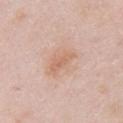A female patient about 65 years old. On the right upper arm. A 15 mm close-up tile from a total-body photography series done for melanoma screening. Measured at roughly 4.5 mm in maximum diameter. Captured under white-light illumination.